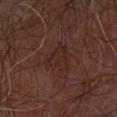Notes:
- workup · no biopsy performed (imaged during a skin exam)
- patient · male, approximately 65 years of age
- lesion diameter · ≈3.5 mm
- anatomic site · the left forearm
- image source · ~15 mm crop, total-body skin-cancer survey